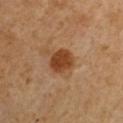notes: no biopsy performed (imaged during a skin exam)
site: the right upper arm
patient: male, approximately 50 years of age
tile lighting: cross-polarized
automated lesion analysis: an outline eccentricity of about 0.45 (0 = round, 1 = elongated); a lesion color around L≈42 a*≈23 b*≈36 in CIELAB and a lesion-to-skin contrast of about 10 (normalized; higher = more distinct); a border-irregularity index near 1.5/10 and internal color variation of about 2.5 on a 0–10 scale; a detector confidence of about 100 out of 100 that the crop contains a lesion
lesion diameter: ≈3 mm
acquisition: total-body-photography crop, ~15 mm field of view